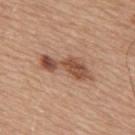| field | value |
|---|---|
| image-analysis metrics | an area of roughly 11 mm² and two-axis asymmetry of about 0.4; roughly 13 lightness units darker than nearby skin and a normalized lesion–skin contrast near 9; a border-irregularity rating of about 5.5/10, a within-lesion color-variation index near 5/10, and radial color variation of about 1.5; an automated nevus-likeness rating near 75 out of 100 and a lesion-detection confidence of about 100/100 |
| site | the upper back |
| lighting | white-light illumination |
| diameter | ≈6.5 mm |
| patient | male, aged approximately 65 |
| image | 15 mm crop, total-body photography |